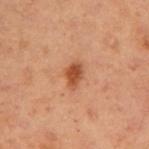Notes:
– biopsy status — catalogued during a skin exam; not biopsied
– tile lighting — cross-polarized illumination
– diameter — about 3 mm
– image — total-body-photography crop, ~15 mm field of view
– subject — female, aged 53–57
– anatomic site — the left upper arm